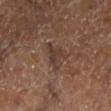Q: Was a biopsy performed?
A: catalogued during a skin exam; not biopsied
Q: Where on the body is the lesion?
A: the left lower leg
Q: What lighting was used for the tile?
A: cross-polarized
Q: How was this image acquired?
A: ~15 mm tile from a whole-body skin photo
Q: Who is the patient?
A: aged 63 to 67
Q: What is the lesion's diameter?
A: ~4 mm (longest diameter)